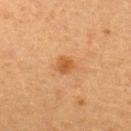Q: Was this lesion biopsied?
A: imaged on a skin check; not biopsied
Q: What kind of image is this?
A: ~15 mm tile from a whole-body skin photo
Q: What is the anatomic site?
A: the upper back
Q: How large is the lesion?
A: ≈2.5 mm
Q: Patient demographics?
A: female, roughly 55 years of age